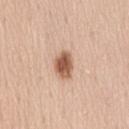This lesion was catalogued during total-body skin photography and was not selected for biopsy. From the back. The patient is a female roughly 40 years of age. A close-up tile cropped from a whole-body skin photograph, about 15 mm across.